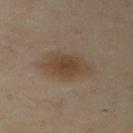Clinical impression: This lesion was catalogued during total-body skin photography and was not selected for biopsy. Image and clinical context: From the arm. Imaged with cross-polarized lighting. The lesion's longest dimension is about 6 mm. Cropped from a total-body skin-imaging series; the visible field is about 15 mm. A male patient, roughly 50 years of age.